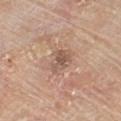Impression: This lesion was catalogued during total-body skin photography and was not selected for biopsy. Clinical summary: A lesion tile, about 15 mm wide, cut from a 3D total-body photograph. Measured at roughly 3 mm in maximum diameter. The lesion is on the chest. A male subject aged 78 to 82.